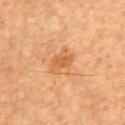Part of a total-body skin-imaging series; this lesion was reviewed on a skin check and was not flagged for biopsy. The tile uses cross-polarized illumination. The subject is a male in their mid- to late 60s. The lesion is located on the chest. Approximately 3.5 mm at its widest. A roughly 15 mm field-of-view crop from a total-body skin photograph.